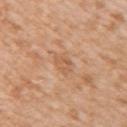Clinical impression: The lesion was tiled from a total-body skin photograph and was not biopsied. Acquisition and patient details: A 15 mm close-up extracted from a 3D total-body photography capture. Automated image analysis of the tile measured a lesion color around L≈59 a*≈21 b*≈35 in CIELAB, roughly 7 lightness units darker than nearby skin, and a normalized lesion–skin contrast near 5. And it measured radial color variation of about 1. A female subject, about 40 years old. The lesion is located on the right upper arm. Captured under white-light illumination. The recorded lesion diameter is about 3 mm.